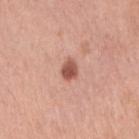<tbp_lesion>
<biopsy_status>not biopsied; imaged during a skin examination</biopsy_status>
<site>right upper arm</site>
<automated_metrics>
  <area_mm2_approx>4.0</area_mm2_approx>
  <eccentricity>0.65</eccentricity>
  <cielab_L>54</cielab_L>
  <cielab_a>25</cielab_a>
  <cielab_b>28</cielab_b>
  <vs_skin_darker_L>15.0</vs_skin_darker_L>
  <vs_skin_contrast_norm>9.5</vs_skin_contrast_norm>
  <border_irregularity_0_10>2.0</border_irregularity_0_10>
</automated_metrics>
<lesion_size>
  <long_diameter_mm_approx>2.5</long_diameter_mm_approx>
</lesion_size>
<image>
  <source>total-body photography crop</source>
  <field_of_view_mm>15</field_of_view_mm>
</image>
<patient>
  <sex>female</sex>
  <age_approx>60</age_approx>
</patient>
</tbp_lesion>